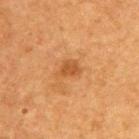illumination = cross-polarized illumination | image = ~15 mm crop, total-body skin-cancer survey | size = ~2.5 mm (longest diameter) | subject = male, roughly 75 years of age | site = the upper back.